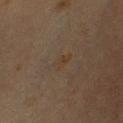  biopsy_status: not biopsied; imaged during a skin examination
  patient:
    sex: male
    age_approx: 35
  lesion_size:
    long_diameter_mm_approx: 3.0
  image:
    source: total-body photography crop
    field_of_view_mm: 15
  site: chest
  automated_metrics:
    cielab_L: 32
    cielab_a: 12
    cielab_b: 24
    vs_skin_darker_L: 3.0